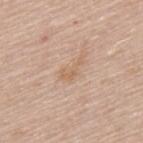{
  "patient": {
    "sex": "male",
    "age_approx": 65
  },
  "site": "upper back",
  "lesion_size": {
    "long_diameter_mm_approx": 3.0
  },
  "image": {
    "source": "total-body photography crop",
    "field_of_view_mm": 15
  }
}